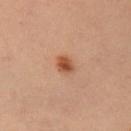Q: Was a biopsy performed?
A: imaged on a skin check; not biopsied
Q: How large is the lesion?
A: ~2.5 mm (longest diameter)
Q: What is the anatomic site?
A: the right upper arm
Q: What kind of image is this?
A: ~15 mm crop, total-body skin-cancer survey
Q: What are the patient's age and sex?
A: male, in their mid-50s
Q: Illumination type?
A: cross-polarized